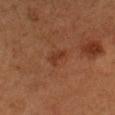workup: total-body-photography surveillance lesion; no biopsy | diameter: ≈2.5 mm | illumination: cross-polarized illumination | image source: ~15 mm tile from a whole-body skin photo | automated metrics: border irregularity of about 4 on a 0–10 scale and a within-lesion color-variation index near 0/10; a nevus-likeness score of about 35/100 and a lesion-detection confidence of about 100/100 | anatomic site: the head or neck | subject: female, aged 38 to 42.